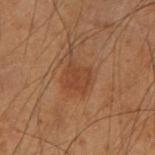| feature | finding |
|---|---|
| notes | imaged on a skin check; not biopsied |
| illumination | cross-polarized illumination |
| location | the arm |
| subject | male, about 55 years old |
| automated metrics | a normalized lesion–skin contrast near 5.5 |
| size | about 3 mm |
| image | ~15 mm crop, total-body skin-cancer survey |